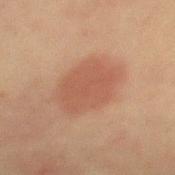Captured during whole-body skin photography for melanoma surveillance; the lesion was not biopsied. Approximately 6.5 mm at its widest. This image is a 15 mm lesion crop taken from a total-body photograph. The lesion is on the mid back. This is a cross-polarized tile. A male patient, aged approximately 60.